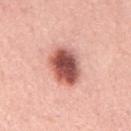Impression: The lesion was tiled from a total-body skin photograph and was not biopsied. Context: The lesion is on the back. Captured under white-light illumination. A 15 mm close-up tile from a total-body photography series done for melanoma screening. The lesion-visualizer software estimated a shape-asymmetry score of about 0.15 (0 = symmetric). It also reported a mean CIELAB color near L≈55 a*≈29 b*≈27 and about 21 CIELAB-L* units darker than the surrounding skin. The analysis additionally found a classifier nevus-likeness of about 100/100 and a detector confidence of about 100 out of 100 that the crop contains a lesion. A male patient, aged 58 to 62. Approximately 5 mm at its widest.